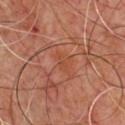Clinical summary: On the chest. A 15 mm crop from a total-body photograph taken for skin-cancer surveillance. Measured at roughly 2.5 mm in maximum diameter. A male patient about 70 years old. Imaged with cross-polarized lighting.